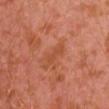acquisition=~15 mm tile from a whole-body skin photo | lesion size=about 3 mm | patient=male, about 30 years old | location=the left arm.